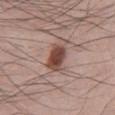- biopsy status · catalogued during a skin exam; not biopsied
- location · the front of the torso
- patient · male, approximately 25 years of age
- illumination · white-light
- imaging modality · 15 mm crop, total-body photography